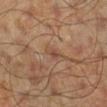{"biopsy_status": "not biopsied; imaged during a skin examination", "automated_metrics": {"cielab_L": 47, "cielab_a": 20, "cielab_b": 29, "vs_skin_darker_L": 7.0, "vs_skin_contrast_norm": 5.5, "border_irregularity_0_10": 2.5, "color_variation_0_10": 0.0, "peripheral_color_asymmetry": 0.0}, "image": {"source": "total-body photography crop", "field_of_view_mm": 15}, "lighting": "cross-polarized", "lesion_size": {"long_diameter_mm_approx": 2.5}, "patient": {"sex": "male", "age_approx": 45}, "site": "right lower leg"}